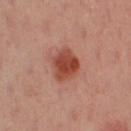The patient is a female about 40 years old.
On the left leg.
The tile uses cross-polarized illumination.
A 15 mm close-up extracted from a 3D total-body photography capture.
Longest diameter approximately 4 mm.
Automated image analysis of the tile measured a mean CIELAB color near L≈47 a*≈28 b*≈31 and roughly 13 lightness units darker than nearby skin. And it measured border irregularity of about 1.5 on a 0–10 scale, a within-lesion color-variation index near 4/10, and a peripheral color-asymmetry measure near 1.